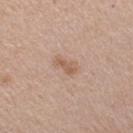Clinical impression:
The lesion was tiled from a total-body skin photograph and was not biopsied.